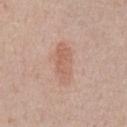Findings:
* workup — catalogued during a skin exam; not biopsied
* subject — male, aged 53 to 57
* lighting — white-light
* site — the chest
* diameter — about 5 mm
* acquisition — 15 mm crop, total-body photography